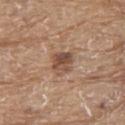Recorded during total-body skin imaging; not selected for excision or biopsy. On the upper back. This image is a 15 mm lesion crop taken from a total-body photograph. Approximately 3 mm at its widest. A male patient in their 80s. This is a white-light tile. Automated tile analysis of the lesion measured an area of roughly 6.5 mm² and a shape-asymmetry score of about 0.2 (0 = symmetric). The analysis additionally found an average lesion color of about L≈49 a*≈18 b*≈28 (CIELAB), a lesion–skin lightness drop of about 11, and a lesion-to-skin contrast of about 8 (normalized; higher = more distinct). And it measured radial color variation of about 2.5. The software also gave a classifier nevus-likeness of about 30/100 and a lesion-detection confidence of about 100/100.